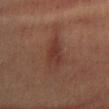{"biopsy_status": "not biopsied; imaged during a skin examination", "patient": {"sex": "male", "age_approx": 55}, "image": {"source": "total-body photography crop", "field_of_view_mm": 15}, "site": "abdomen", "lesion_size": {"long_diameter_mm_approx": 4.5}, "lighting": "cross-polarized"}